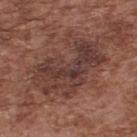Q: Is there a histopathology result?
A: total-body-photography surveillance lesion; no biopsy
Q: What is the imaging modality?
A: 15 mm crop, total-body photography
Q: What is the lesion's diameter?
A: ≈8 mm
Q: What lighting was used for the tile?
A: white-light illumination
Q: Who is the patient?
A: male, roughly 75 years of age
Q: Where on the body is the lesion?
A: the upper back